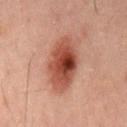Assessment: No biopsy was performed on this lesion — it was imaged during a full skin examination and was not determined to be concerning. Background: On the mid back. The subject is a male in their 60s. Cropped from a total-body skin-imaging series; the visible field is about 15 mm.